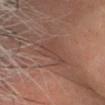• biopsy status: total-body-photography surveillance lesion; no biopsy
• diameter: ~4.5 mm (longest diameter)
• anatomic site: the head or neck
• illumination: cross-polarized
• acquisition: 15 mm crop, total-body photography
• automated lesion analysis: an area of roughly 8.5 mm², an eccentricity of roughly 0.8, and a shape-asymmetry score of about 0.5 (0 = symmetric); a lesion color around L≈44 a*≈20 b*≈25 in CIELAB and a normalized lesion–skin contrast near 4.5; internal color variation of about 1.5 on a 0–10 scale and a peripheral color-asymmetry measure near 0.5; a classifier nevus-likeness of about 0/100 and a detector confidence of about 85 out of 100 that the crop contains a lesion
• patient: male, in their mid-60s